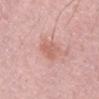Captured during whole-body skin photography for melanoma surveillance; the lesion was not biopsied. A 15 mm crop from a total-body photograph taken for skin-cancer surveillance. This is a white-light tile. An algorithmic analysis of the crop reported an average lesion color of about L≈62 a*≈25 b*≈27 (CIELAB), a lesion–skin lightness drop of about 8, and a normalized border contrast of about 6. It also reported a nevus-likeness score of about 10/100 and a detector confidence of about 100 out of 100 that the crop contains a lesion. The lesion is on the arm. A male subject, in their mid-20s. Longest diameter approximately 3.5 mm.